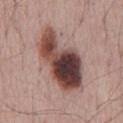| key | value |
|---|---|
| follow-up | catalogued during a skin exam; not biopsied |
| image-analysis metrics | a lesion area of about 27 mm², an eccentricity of roughly 0.9, and two-axis asymmetry of about 0.25; a lesion–skin lightness drop of about 20 and a lesion-to-skin contrast of about 14 (normalized; higher = more distinct); an automated nevus-likeness rating near 15 out of 100 and a detector confidence of about 100 out of 100 that the crop contains a lesion |
| anatomic site | the back |
| image | 15 mm crop, total-body photography |
| lighting | white-light illumination |
| size | ~8.5 mm (longest diameter) |
| subject | male, about 65 years old |